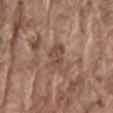No biopsy was performed on this lesion — it was imaged during a full skin examination and was not determined to be concerning. The tile uses white-light illumination. Cropped from a total-body skin-imaging series; the visible field is about 15 mm. From the back. About 3 mm across. Automated image analysis of the tile measured an area of roughly 5.5 mm², a shape eccentricity near 0.8, and a symmetry-axis asymmetry near 0.2. The software also gave about 8 CIELAB-L* units darker than the surrounding skin and a lesion-to-skin contrast of about 6 (normalized; higher = more distinct). It also reported border irregularity of about 2.5 on a 0–10 scale, internal color variation of about 4 on a 0–10 scale, and a peripheral color-asymmetry measure near 1.5. A male patient, in their 80s.